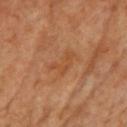Imaged during a routine full-body skin examination; the lesion was not biopsied and no histopathology is available.
From the chest.
A 15 mm close-up extracted from a 3D total-body photography capture.
A female subject.